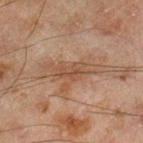Notes:
* notes — total-body-photography surveillance lesion; no biopsy
* tile lighting — cross-polarized
* image — 15 mm crop, total-body photography
* patient — male, aged approximately 45
* automated lesion analysis — internal color variation of about 1.5 on a 0–10 scale
* location — the right lower leg